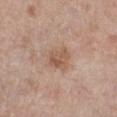Q: Where on the body is the lesion?
A: the leg
Q: What kind of image is this?
A: ~15 mm crop, total-body skin-cancer survey
Q: Patient demographics?
A: female, in their mid- to late 50s
Q: What is the lesion's diameter?
A: ≈3 mm